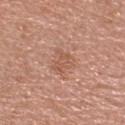Assessment: Part of a total-body skin-imaging series; this lesion was reviewed on a skin check and was not flagged for biopsy. Background: A male subject aged 58 to 62. The lesion is on the head or neck. This is a white-light tile. Approximately 3.5 mm at its widest. A close-up tile cropped from a whole-body skin photograph, about 15 mm across.